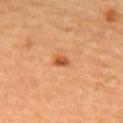Impression:
Recorded during total-body skin imaging; not selected for excision or biopsy.
Acquisition and patient details:
The recorded lesion diameter is about 2 mm. A female patient, about 30 years old. An algorithmic analysis of the crop reported an area of roughly 2.5 mm², a shape eccentricity near 0.7, and a shape-asymmetry score of about 0.3 (0 = symmetric). And it measured peripheral color asymmetry of about 1.5. The software also gave a nevus-likeness score of about 90/100. Located on the upper back. A close-up tile cropped from a whole-body skin photograph, about 15 mm across.